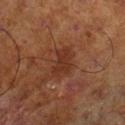Recorded during total-body skin imaging; not selected for excision or biopsy. A 15 mm close-up tile from a total-body photography series done for melanoma screening. From the right lower leg. The subject is a male about 70 years old.